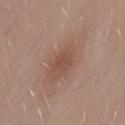Assessment:
Imaged during a routine full-body skin examination; the lesion was not biopsied and no histopathology is available.
Image and clinical context:
A 15 mm crop from a total-body photograph taken for skin-cancer surveillance. Measured at roughly 3 mm in maximum diameter. This is a white-light tile. The lesion is located on the back. A male patient approximately 30 years of age.